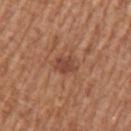Q: Who is the patient?
A: male, aged around 65
Q: What lighting was used for the tile?
A: white-light
Q: Lesion location?
A: the arm
Q: How was this image acquired?
A: total-body-photography crop, ~15 mm field of view
Q: Lesion size?
A: ~2.5 mm (longest diameter)